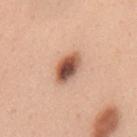Q: Was a biopsy performed?
A: no biopsy performed (imaged during a skin exam)